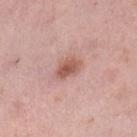Recorded during total-body skin imaging; not selected for excision or biopsy.
The recorded lesion diameter is about 3.5 mm.
A lesion tile, about 15 mm wide, cut from a 3D total-body photograph.
The subject is a female in their 50s.
The lesion is located on the left lower leg.
Captured under white-light illumination.